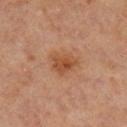Impression: The lesion was tiled from a total-body skin photograph and was not biopsied. Acquisition and patient details: The lesion's longest dimension is about 3.5 mm. A 15 mm close-up extracted from a 3D total-body photography capture. An algorithmic analysis of the crop reported an eccentricity of roughly 0.55 and a symmetry-axis asymmetry near 0.2. It also reported a detector confidence of about 100 out of 100 that the crop contains a lesion. The subject is a male roughly 60 years of age. The lesion is located on the right lower leg.